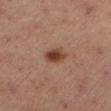patient:
  sex: male
  age_approx: 55
image:
  source: total-body photography crop
  field_of_view_mm: 15
site: left lower leg